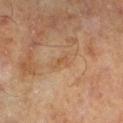The lesion was photographed on a routine skin check and not biopsied; there is no pathology result.
The lesion is located on the leg.
A roughly 15 mm field-of-view crop from a total-body skin photograph.
A male patient aged around 65.